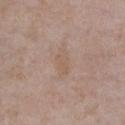Imaged during a routine full-body skin examination; the lesion was not biopsied and no histopathology is available. A female patient approximately 50 years of age. Captured under white-light illumination. Longest diameter approximately 3.5 mm. A close-up tile cropped from a whole-body skin photograph, about 15 mm across. The lesion is located on the chest.